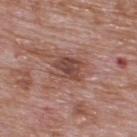<record>
  <biopsy_status>not biopsied; imaged during a skin examination</biopsy_status>
  <site>upper back</site>
  <image>
    <source>total-body photography crop</source>
    <field_of_view_mm>15</field_of_view_mm>
  </image>
  <patient>
    <sex>male</sex>
    <age_approx>70</age_approx>
  </patient>
</record>